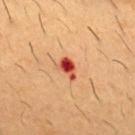No biopsy was performed on this lesion — it was imaged during a full skin examination and was not determined to be concerning.
A male patient, aged approximately 55.
From the chest.
Imaged with cross-polarized lighting.
A close-up tile cropped from a whole-body skin photograph, about 15 mm across.